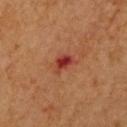Captured during whole-body skin photography for melanoma surveillance; the lesion was not biopsied. The lesion-visualizer software estimated a footprint of about 4 mm², an eccentricity of roughly 0.8, and a shape-asymmetry score of about 0.35 (0 = symmetric). The analysis additionally found a border-irregularity rating of about 3.5/10, internal color variation of about 2.5 on a 0–10 scale, and a peripheral color-asymmetry measure near 0.5. The software also gave an automated nevus-likeness rating near 0 out of 100 and lesion-presence confidence of about 100/100. A male patient aged around 65. The lesion is on the left upper arm. A 15 mm crop from a total-body photograph taken for skin-cancer surveillance. Imaged with cross-polarized lighting. Approximately 3 mm at its widest.